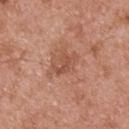notes: no biopsy performed (imaged during a skin exam)
size: ≈3 mm
patient: male, aged around 55
image-analysis metrics: border irregularity of about 5.5 on a 0–10 scale, a color-variation rating of about 1/10, and a peripheral color-asymmetry measure near 0.5
site: the upper back
image source: ~15 mm tile from a whole-body skin photo